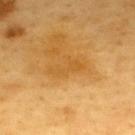{"biopsy_status": "not biopsied; imaged during a skin examination", "automated_metrics": {"area_mm2_approx": 4.5, "eccentricity": 0.9, "cielab_L": 56, "cielab_a": 22, "cielab_b": 50, "vs_skin_contrast_norm": 5.0, "border_irregularity_0_10": 8.5, "color_variation_0_10": 0.5, "peripheral_color_asymmetry": 0.0, "nevus_likeness_0_100": 10}, "patient": {"sex": "male", "age_approx": 60}, "image": {"source": "total-body photography crop", "field_of_view_mm": 15}, "site": "back", "lighting": "cross-polarized", "lesion_size": {"long_diameter_mm_approx": 4.0}}